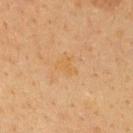biopsy status: catalogued during a skin exam; not biopsied
subject: female, aged approximately 40
imaging modality: ~15 mm tile from a whole-body skin photo
body site: the upper back
tile lighting: cross-polarized
automated metrics: a footprint of about 3.5 mm², a shape eccentricity near 0.7, and a shape-asymmetry score of about 0.45 (0 = symmetric)
size: ≈2.5 mm